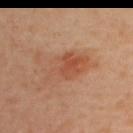Located on the upper back.
The recorded lesion diameter is about 6.5 mm.
Cropped from a whole-body photographic skin survey; the tile spans about 15 mm.
A female subject, aged approximately 40.